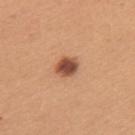No biopsy was performed on this lesion — it was imaged during a full skin examination and was not determined to be concerning.
A region of skin cropped from a whole-body photographic capture, roughly 15 mm wide.
The lesion's longest dimension is about 3 mm.
On the upper back.
This is a white-light tile.
The total-body-photography lesion software estimated a mean CIELAB color near L≈50 a*≈24 b*≈33, a lesion–skin lightness drop of about 17, and a normalized lesion–skin contrast near 11.
A female subject, roughly 30 years of age.